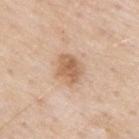diameter — ~3.5 mm (longest diameter) | acquisition — total-body-photography crop, ~15 mm field of view | location — the upper back | lighting — white-light | patient — male, in their 80s.